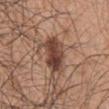Clinical impression:
The lesion was photographed on a routine skin check and not biopsied; there is no pathology result.
Acquisition and patient details:
A 15 mm close-up extracted from a 3D total-body photography capture. Captured under white-light illumination. The lesion's longest dimension is about 4.5 mm. Located on the right upper arm. A male patient, aged 43 to 47. The lesion-visualizer software estimated a lesion area of about 11 mm², an outline eccentricity of about 0.75 (0 = round, 1 = elongated), and a symmetry-axis asymmetry near 0.2.